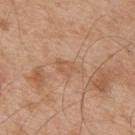follow-up: no biopsy performed (imaged during a skin exam); anatomic site: the back; acquisition: ~15 mm crop, total-body skin-cancer survey; lesion diameter: ~3.5 mm (longest diameter); patient: male, aged around 55; tile lighting: white-light.